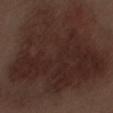Impression:
This lesion was catalogued during total-body skin photography and was not selected for biopsy.
Image and clinical context:
This is a white-light tile. This image is a 15 mm lesion crop taken from a total-body photograph. A male subject approximately 70 years of age. Located on the right forearm.